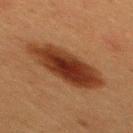Clinical impression: This lesion was catalogued during total-body skin photography and was not selected for biopsy. Background: The tile uses cross-polarized illumination. The recorded lesion diameter is about 8 mm. Automated tile analysis of the lesion measured a border-irregularity rating of about 2.5/10, a color-variation rating of about 5/10, and radial color variation of about 1.5. The patient is a male roughly 40 years of age. On the mid back. This image is a 15 mm lesion crop taken from a total-body photograph.